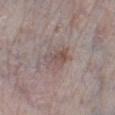Captured during whole-body skin photography for melanoma surveillance; the lesion was not biopsied. Automated image analysis of the tile measured a footprint of about 5 mm², an outline eccentricity of about 0.8 (0 = round, 1 = elongated), and two-axis asymmetry of about 0.4. The software also gave an automated nevus-likeness rating near 15 out of 100 and a detector confidence of about 95 out of 100 that the crop contains a lesion. A 15 mm crop from a total-body photograph taken for skin-cancer surveillance. Captured under white-light illumination. A male patient, aged around 75. The lesion is located on the right lower leg. Measured at roughly 3.5 mm in maximum diameter.